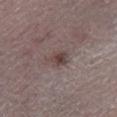{"biopsy_status": "not biopsied; imaged during a skin examination", "patient": {"sex": "male", "age_approx": 75}, "image": {"source": "total-body photography crop", "field_of_view_mm": 15}, "site": "left lower leg", "lighting": "white-light"}